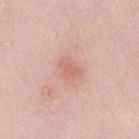Clinical impression: The lesion was photographed on a routine skin check and not biopsied; there is no pathology result. Clinical summary: A 15 mm close-up tile from a total-body photography series done for melanoma screening. Longest diameter approximately 3 mm. Located on the back. Captured under white-light illumination. The lesion-visualizer software estimated an area of roughly 4 mm², a shape eccentricity near 0.75, and a symmetry-axis asymmetry near 0.4. The software also gave a nevus-likeness score of about 20/100 and a detector confidence of about 100 out of 100 that the crop contains a lesion. The patient is a male aged 23 to 27.